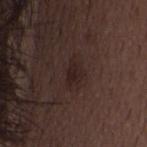Clinical impression: Captured during whole-body skin photography for melanoma surveillance; the lesion was not biopsied. Clinical summary: A region of skin cropped from a whole-body photographic capture, roughly 15 mm wide. About 3 mm across. The patient is a male aged 48–52. Imaged with white-light lighting. On the head or neck. An algorithmic analysis of the crop reported a lesion-detection confidence of about 100/100.